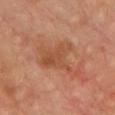Findings:
• follow-up — no biopsy performed (imaged during a skin exam)
• diameter — about 6 mm
• illumination — cross-polarized
• image — ~15 mm tile from a whole-body skin photo
• patient — male, roughly 65 years of age
• site — the chest